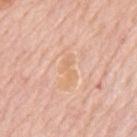Imaged during a routine full-body skin examination; the lesion was not biopsied and no histopathology is available.
The patient is a male roughly 80 years of age.
From the mid back.
This image is a 15 mm lesion crop taken from a total-body photograph.